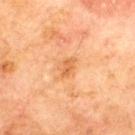<lesion>
<biopsy_status>not biopsied; imaged during a skin examination</biopsy_status>
<image>
  <source>total-body photography crop</source>
  <field_of_view_mm>15</field_of_view_mm>
</image>
<patient>
  <sex>male</sex>
  <age_approx>70</age_approx>
</patient>
<site>upper back</site>
</lesion>